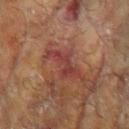Part of a total-body skin-imaging series; this lesion was reviewed on a skin check and was not flagged for biopsy.
A female patient, aged 78–82.
The lesion is located on the left arm.
A roughly 15 mm field-of-view crop from a total-body skin photograph.